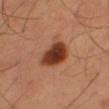Findings:
• notes · catalogued during a skin exam; not biopsied
• diameter · ~4.5 mm (longest diameter)
• location · the leg
• lighting · cross-polarized illumination
• acquisition · total-body-photography crop, ~15 mm field of view
• subject · male, approximately 50 years of age